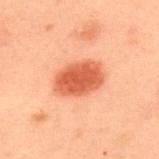This lesion was catalogued during total-body skin photography and was not selected for biopsy. The lesion is on the upper back. A region of skin cropped from a whole-body photographic capture, roughly 15 mm wide. Approximately 5 mm at its widest. A male patient, aged around 55.